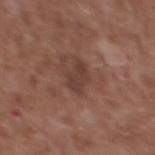– follow-up · imaged on a skin check; not biopsied
– body site · the upper back
– imaging modality · total-body-photography crop, ~15 mm field of view
– lesion size · ≈3 mm
– image-analysis metrics · a lesion area of about 5.5 mm², a shape eccentricity near 0.7, and a symmetry-axis asymmetry near 0.3; about 7 CIELAB-L* units darker than the surrounding skin and a normalized border contrast of about 6.5
– subject · male, approximately 45 years of age
– illumination · white-light illumination